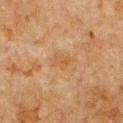Background: Cropped from a total-body skin-imaging series; the visible field is about 15 mm. Located on the chest. A male subject, roughly 80 years of age. Imaged with cross-polarized lighting. The total-body-photography lesion software estimated a lesion area of about 3.5 mm², an outline eccentricity of about 0.75 (0 = round, 1 = elongated), and a symmetry-axis asymmetry near 0.3. The software also gave a lesion color around L≈44 a*≈17 b*≈33 in CIELAB, a lesion–skin lightness drop of about 5, and a lesion-to-skin contrast of about 5.5 (normalized; higher = more distinct). It also reported an automated nevus-likeness rating near 0 out of 100 and a lesion-detection confidence of about 100/100. About 2.5 mm across.